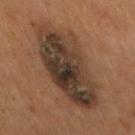Imaged during a routine full-body skin examination; the lesion was not biopsied and no histopathology is available. A male patient, aged 53–57. Located on the mid back. A roughly 15 mm field-of-view crop from a total-body skin photograph.